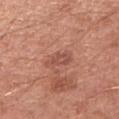notes: catalogued during a skin exam; not biopsied
location: the left upper arm
image: ~15 mm tile from a whole-body skin photo
illumination: white-light illumination
diameter: ~3 mm (longest diameter)
subject: male, roughly 55 years of age